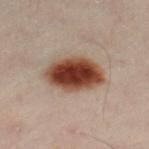notes = no biopsy performed (imaged during a skin exam)
location = the leg
tile lighting = cross-polarized
imaging modality = ~15 mm tile from a whole-body skin photo
patient = male, in their 50s